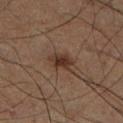<tbp_lesion>
<biopsy_status>not biopsied; imaged during a skin examination</biopsy_status>
<lighting>cross-polarized</lighting>
<image>
  <source>total-body photography crop</source>
  <field_of_view_mm>15</field_of_view_mm>
</image>
<patient>
  <sex>male</sex>
  <age_approx>60</age_approx>
</patient>
<site>right lower leg</site>
</tbp_lesion>